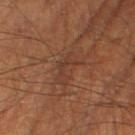Image and clinical context: A region of skin cropped from a whole-body photographic capture, roughly 15 mm wide. The lesion is on the right thigh. The tile uses cross-polarized illumination. The recorded lesion diameter is about 4 mm. The patient is a male approximately 60 years of age.